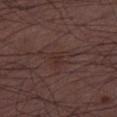Q: Was a biopsy performed?
A: total-body-photography surveillance lesion; no biopsy
Q: What is the anatomic site?
A: the right thigh
Q: What did automated image analysis measure?
A: border irregularity of about 5 on a 0–10 scale, internal color variation of about 1.5 on a 0–10 scale, and peripheral color asymmetry of about 0.5
Q: What is the imaging modality?
A: ~15 mm crop, total-body skin-cancer survey
Q: What are the patient's age and sex?
A: male, aged 48–52
Q: What lighting was used for the tile?
A: white-light illumination
Q: Lesion size?
A: about 3 mm